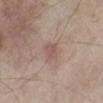Case summary:
- follow-up · no biopsy performed (imaged during a skin exam)
- subject · male, approximately 60 years of age
- size · ≈2.5 mm
- illumination · white-light illumination
- image · total-body-photography crop, ~15 mm field of view
- site · the left lower leg
- image-analysis metrics · a classifier nevus-likeness of about 10/100